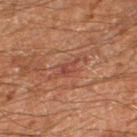Part of a total-body skin-imaging series; this lesion was reviewed on a skin check and was not flagged for biopsy.
The total-body-photography lesion software estimated an outline eccentricity of about 0.85 (0 = round, 1 = elongated) and a symmetry-axis asymmetry near 0.35. The software also gave border irregularity of about 4 on a 0–10 scale and radial color variation of about 0.5. The analysis additionally found a detector confidence of about 75 out of 100 that the crop contains a lesion.
The lesion's longest dimension is about 3 mm.
The lesion is on the right thigh.
The subject is a male roughly 60 years of age.
A 15 mm crop from a total-body photograph taken for skin-cancer surveillance.